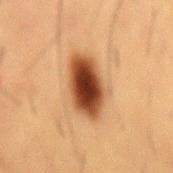  biopsy_status: not biopsied; imaged during a skin examination
  image:
    source: total-body photography crop
    field_of_view_mm: 15
  lesion_size:
    long_diameter_mm_approx: 6.0
  site: abdomen
  patient:
    sex: male
    age_approx: 55